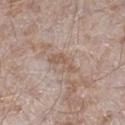The lesion was photographed on a routine skin check and not biopsied; there is no pathology result. A 15 mm close-up tile from a total-body photography series done for melanoma screening. This is a white-light tile. The lesion's longest dimension is about 3.5 mm. The lesion is on the right lower leg. Automated image analysis of the tile measured a lesion color around L≈56 a*≈16 b*≈26 in CIELAB. A male subject in their mid-40s.